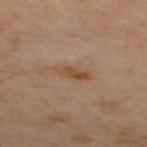{
  "biopsy_status": "not biopsied; imaged during a skin examination",
  "site": "mid back",
  "lighting": "cross-polarized",
  "image": {
    "source": "total-body photography crop",
    "field_of_view_mm": 15
  },
  "automated_metrics": {
    "area_mm2_approx": 4.5,
    "eccentricity": 0.9,
    "cielab_L": 49,
    "cielab_a": 19,
    "cielab_b": 34,
    "vs_skin_darker_L": 8.0,
    "vs_skin_contrast_norm": 7.5,
    "nevus_likeness_0_100": 15,
    "lesion_detection_confidence_0_100": 100
  },
  "lesion_size": {
    "long_diameter_mm_approx": 3.5
  }
}